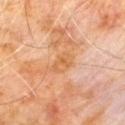Assessment:
Recorded during total-body skin imaging; not selected for excision or biopsy.
Clinical summary:
Imaged with cross-polarized lighting. The lesion is on the chest. About 3 mm across. A male patient roughly 60 years of age. The lesion-visualizer software estimated roughly 6 lightness units darker than nearby skin and a lesion-to-skin contrast of about 5.5 (normalized; higher = more distinct). It also reported border irregularity of about 4.5 on a 0–10 scale, a within-lesion color-variation index near 2/10, and a peripheral color-asymmetry measure near 0.5. It also reported an automated nevus-likeness rating near 0 out of 100. A 15 mm close-up tile from a total-body photography series done for melanoma screening.